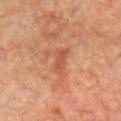Q: Is there a histopathology result?
A: no biopsy performed (imaged during a skin exam)
Q: Illumination type?
A: cross-polarized illumination
Q: What are the patient's age and sex?
A: male, approximately 75 years of age
Q: What is the lesion's diameter?
A: about 3.5 mm
Q: Where on the body is the lesion?
A: the front of the torso
Q: What did automated image analysis measure?
A: a footprint of about 4.5 mm², an eccentricity of roughly 0.9, and a symmetry-axis asymmetry near 0.35; a lesion–skin lightness drop of about 7 and a lesion-to-skin contrast of about 5.5 (normalized; higher = more distinct); border irregularity of about 3.5 on a 0–10 scale, a within-lesion color-variation index near 2/10, and peripheral color asymmetry of about 0.5; a nevus-likeness score of about 0/100 and a lesion-detection confidence of about 100/100
Q: What kind of image is this?
A: total-body-photography crop, ~15 mm field of view